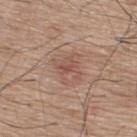Automated tile analysis of the lesion measured a shape eccentricity near 0.6 and a symmetry-axis asymmetry near 0.3. The analysis additionally found an average lesion color of about L≈53 a*≈19 b*≈26 (CIELAB). The analysis additionally found a border-irregularity rating of about 3/10, internal color variation of about 5 on a 0–10 scale, and radial color variation of about 1.5. The analysis additionally found an automated nevus-likeness rating near 0 out of 100. The patient is a male approximately 75 years of age. Approximately 4 mm at its widest. The lesion is on the upper back. A 15 mm close-up tile from a total-body photography series done for melanoma screening.